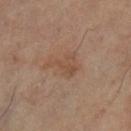Q: Was a biopsy performed?
A: no biopsy performed (imaged during a skin exam)
Q: How large is the lesion?
A: ~4 mm (longest diameter)
Q: Automated lesion metrics?
A: a footprint of about 6 mm², an eccentricity of roughly 0.8, and a shape-asymmetry score of about 0.65 (0 = symmetric); an average lesion color of about L≈49 a*≈18 b*≈30 (CIELAB), roughly 6 lightness units darker than nearby skin, and a normalized lesion–skin contrast near 5.5; a classifier nevus-likeness of about 0/100 and lesion-presence confidence of about 100/100
Q: Lesion location?
A: the leg
Q: Patient demographics?
A: female, aged 58 to 62
Q: How was the tile lit?
A: cross-polarized illumination
Q: What is the imaging modality?
A: total-body-photography crop, ~15 mm field of view